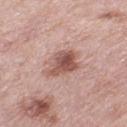– follow-up — total-body-photography surveillance lesion; no biopsy
– imaging modality — 15 mm crop, total-body photography
– patient — female, about 40 years old
– size — about 4 mm
– TBP lesion metrics — internal color variation of about 6 on a 0–10 scale and a peripheral color-asymmetry measure near 1.5; a detector confidence of about 100 out of 100 that the crop contains a lesion
– tile lighting — white-light
– body site — the left thigh